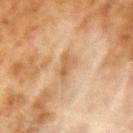| key | value |
|---|---|
| biopsy status | imaged on a skin check; not biopsied |
| lesion diameter | ≈3 mm |
| patient | male, aged around 70 |
| site | the left upper arm |
| imaging modality | 15 mm crop, total-body photography |